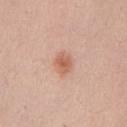biopsy status = no biopsy performed (imaged during a skin exam) | image = 15 mm crop, total-body photography | lesion diameter = ~3 mm (longest diameter) | site = the chest | tile lighting = white-light | subject = female, in their mid- to late 20s.